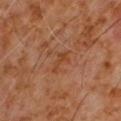  biopsy_status: not biopsied; imaged during a skin examination
  patient:
    sex: male
    age_approx: 60
  image:
    source: total-body photography crop
    field_of_view_mm: 15
  automated_metrics:
    shape_asymmetry: 0.45
    nevus_likeness_0_100: 0
  site: chest
  lighting: cross-polarized
  lesion_size:
    long_diameter_mm_approx: 3.0